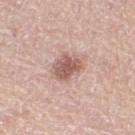Case summary:
* follow-up — imaged on a skin check; not biopsied
* anatomic site — the right thigh
* acquisition — ~15 mm tile from a whole-body skin photo
* subject — female, in their mid- to late 60s